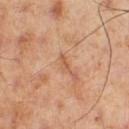Clinical impression: Captured during whole-body skin photography for melanoma surveillance; the lesion was not biopsied. Acquisition and patient details: The total-body-photography lesion software estimated an outline eccentricity of about 0.9 (0 = round, 1 = elongated) and two-axis asymmetry of about 0.4. The software also gave border irregularity of about 4 on a 0–10 scale and peripheral color asymmetry of about 0. Imaged with cross-polarized lighting. A male subject, in their mid-50s. On the right thigh. A close-up tile cropped from a whole-body skin photograph, about 15 mm across.